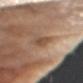No biopsy was performed on this lesion — it was imaged during a full skin examination and was not determined to be concerning.
A 15 mm close-up extracted from a 3D total-body photography capture.
Measured at roughly 2.5 mm in maximum diameter.
The tile uses white-light illumination.
A male patient, aged around 75.
From the chest.